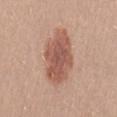biopsy status: total-body-photography surveillance lesion; no biopsy
body site: the lower back
subject: female, approximately 25 years of age
imaging modality: total-body-photography crop, ~15 mm field of view
tile lighting: white-light illumination